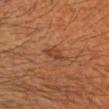Captured during whole-body skin photography for melanoma surveillance; the lesion was not biopsied. A male subject aged approximately 60. The tile uses cross-polarized illumination. About 3 mm across. An algorithmic analysis of the crop reported an area of roughly 3.5 mm² and two-axis asymmetry of about 0.3. And it measured an average lesion color of about L≈39 a*≈22 b*≈32 (CIELAB). And it measured lesion-presence confidence of about 100/100. A 15 mm close-up tile from a total-body photography series done for melanoma screening. From the head or neck.